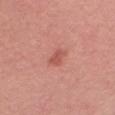{"patient": {"sex": "female", "age_approx": 25}, "site": "chest", "lesion_size": {"long_diameter_mm_approx": 2.5}, "lighting": "white-light", "image": {"source": "total-body photography crop", "field_of_view_mm": 15}}